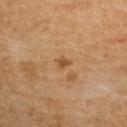Recorded during total-body skin imaging; not selected for excision or biopsy. On the upper back. Automated image analysis of the tile measured a footprint of about 2 mm² and a shape-asymmetry score of about 0.35 (0 = symmetric). The software also gave a border-irregularity index near 3/10, internal color variation of about 1 on a 0–10 scale, and a peripheral color-asymmetry measure near 0.5. The analysis additionally found an automated nevus-likeness rating near 5 out of 100 and lesion-presence confidence of about 100/100. Imaged with cross-polarized lighting. A female subject aged approximately 40. Cropped from a total-body skin-imaging series; the visible field is about 15 mm. The recorded lesion diameter is about 1.5 mm.